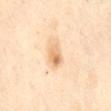Assessment: Recorded during total-body skin imaging; not selected for excision or biopsy. Background: The total-body-photography lesion software estimated a lesion color around L≈76 a*≈19 b*≈41 in CIELAB, a lesion–skin lightness drop of about 12, and a normalized lesion–skin contrast near 7. The analysis additionally found border irregularity of about 2 on a 0–10 scale and radial color variation of about 3. The lesion is located on the mid back. About 3 mm across. A close-up tile cropped from a whole-body skin photograph, about 15 mm across. A female subject, aged around 55.